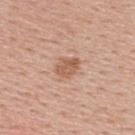Assessment:
Captured during whole-body skin photography for melanoma surveillance; the lesion was not biopsied.
Clinical summary:
A female subject, about 40 years old. The recorded lesion diameter is about 3 mm. A close-up tile cropped from a whole-body skin photograph, about 15 mm across. This is a white-light tile. The lesion is located on the upper back.